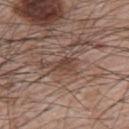workup = no biopsy performed (imaged during a skin exam) | site = the left upper arm | image source = ~15 mm tile from a whole-body skin photo | size = ~2.5 mm (longest diameter) | tile lighting = white-light illumination | patient = male, in their mid-60s.